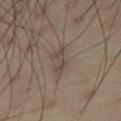Q: Was a biopsy performed?
A: no biopsy performed (imaged during a skin exam)
Q: What is the anatomic site?
A: the right thigh
Q: Who is the patient?
A: male, aged approximately 40
Q: What is the imaging modality?
A: 15 mm crop, total-body photography